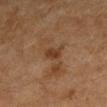Notes:
• workup: imaged on a skin check; not biopsied
• patient: female, in their 60s
• anatomic site: the left upper arm
• TBP lesion metrics: an outline eccentricity of about 0.8 (0 = round, 1 = elongated) and two-axis asymmetry of about 0.25; an average lesion color of about L≈34 a*≈19 b*≈30 (CIELAB), about 8 CIELAB-L* units darker than the surrounding skin, and a lesion-to-skin contrast of about 7.5 (normalized; higher = more distinct); an automated nevus-likeness rating near 0 out of 100 and a lesion-detection confidence of about 100/100
• imaging modality: 15 mm crop, total-body photography